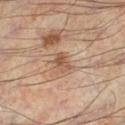Q: Was a biopsy performed?
A: no biopsy performed (imaged during a skin exam)
Q: How was this image acquired?
A: ~15 mm tile from a whole-body skin photo
Q: Who is the patient?
A: male, about 55 years old
Q: Lesion location?
A: the left lower leg
Q: What did automated image analysis measure?
A: a classifier nevus-likeness of about 0/100 and a lesion-detection confidence of about 100/100
Q: Lesion size?
A: ~2.5 mm (longest diameter)
Q: What lighting was used for the tile?
A: cross-polarized illumination